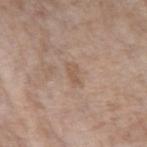| feature | finding |
|---|---|
| notes | catalogued during a skin exam; not biopsied |
| anatomic site | the left forearm |
| patient | female, aged around 85 or older |
| image source | total-body-photography crop, ~15 mm field of view |
| tile lighting | white-light illumination |